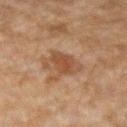biopsy status: imaged on a skin check; not biopsied | image: 15 mm crop, total-body photography | lighting: cross-polarized illumination | subject: male, about 45 years old | lesion diameter: about 3.5 mm | image-analysis metrics: a footprint of about 6 mm², a shape eccentricity near 0.8, and two-axis asymmetry of about 0.25; an average lesion color of about L≈39 a*≈18 b*≈27 (CIELAB), a lesion–skin lightness drop of about 8, and a lesion-to-skin contrast of about 7 (normalized; higher = more distinct); border irregularity of about 2.5 on a 0–10 scale, internal color variation of about 2 on a 0–10 scale, and radial color variation of about 0.5 | body site: the arm.